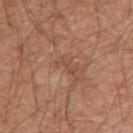Case summary:
– biopsy status: imaged on a skin check; not biopsied
– anatomic site: the right upper arm
– patient: male, aged approximately 55
– image: total-body-photography crop, ~15 mm field of view
– automated lesion analysis: a classifier nevus-likeness of about 0/100 and a lesion-detection confidence of about 100/100